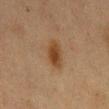Part of a total-body skin-imaging series; this lesion was reviewed on a skin check and was not flagged for biopsy.
The recorded lesion diameter is about 4 mm.
This image is a 15 mm lesion crop taken from a total-body photograph.
Imaged with cross-polarized lighting.
The subject is a female roughly 40 years of age.
The lesion is on the left thigh.
Automated tile analysis of the lesion measured an average lesion color of about L≈38 a*≈17 b*≈31 (CIELAB), a lesion–skin lightness drop of about 9, and a lesion-to-skin contrast of about 8.5 (normalized; higher = more distinct).